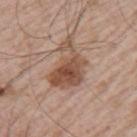Recorded during total-body skin imaging; not selected for excision or biopsy. The subject is a male aged around 65. The lesion's longest dimension is about 6 mm. This is a white-light tile. A 15 mm close-up tile from a total-body photography series done for melanoma screening. The lesion is on the left upper arm.